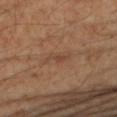A region of skin cropped from a whole-body photographic capture, roughly 15 mm wide.
A female patient aged around 60.
The total-body-photography lesion software estimated a border-irregularity rating of about 6.5/10, a color-variation rating of about 0/10, and radial color variation of about 0.
The tile uses cross-polarized illumination.
From the left forearm.